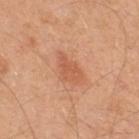Case summary:
- workup: catalogued during a skin exam; not biopsied
- location: the upper back
- image: 15 mm crop, total-body photography
- subject: male, aged 48 to 52
- lighting: white-light illumination